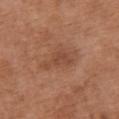This lesion was catalogued during total-body skin photography and was not selected for biopsy. The lesion is located on the chest. A female patient, aged around 65. A roughly 15 mm field-of-view crop from a total-body skin photograph. Imaged with white-light lighting.